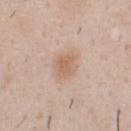The lesion was tiled from a total-body skin photograph and was not biopsied.
From the chest.
Imaged with white-light lighting.
This image is a 15 mm lesion crop taken from a total-body photograph.
The recorded lesion diameter is about 2.5 mm.
A male subject aged 38–42.